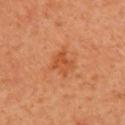Case summary:
* notes · total-body-photography surveillance lesion; no biopsy
* lighting · cross-polarized illumination
* image source · total-body-photography crop, ~15 mm field of view
* subject · female, in their 40s
* automated lesion analysis · an average lesion color of about L≈53 a*≈30 b*≈42 (CIELAB), roughly 8 lightness units darker than nearby skin, and a normalized lesion–skin contrast near 6; a nevus-likeness score of about 60/100 and a lesion-detection confidence of about 100/100
* size · about 3 mm
* location · the right upper arm